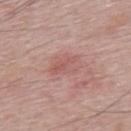Acquisition and patient details:
A male patient in their mid-60s. This image is a 15 mm lesion crop taken from a total-body photograph. The lesion is located on the mid back.